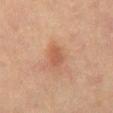Notes:
– notes · total-body-photography surveillance lesion; no biopsy
– imaging modality · 15 mm crop, total-body photography
– location · the mid back
– patient · male, about 65 years old